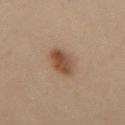Part of a total-body skin-imaging series; this lesion was reviewed on a skin check and was not flagged for biopsy.
Captured under cross-polarized illumination.
Longest diameter approximately 4 mm.
A roughly 15 mm field-of-view crop from a total-body skin photograph.
The patient is a female aged around 30.
From the abdomen.